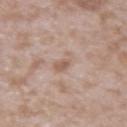Part of a total-body skin-imaging series; this lesion was reviewed on a skin check and was not flagged for biopsy. The tile uses white-light illumination. On the right upper arm. A male patient roughly 45 years of age. Cropped from a whole-body photographic skin survey; the tile spans about 15 mm.